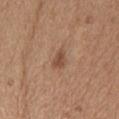Background: The lesion's longest dimension is about 2.5 mm. The tile uses white-light illumination. The lesion-visualizer software estimated a nevus-likeness score of about 75/100 and lesion-presence confidence of about 100/100. A female patient, aged around 70. The lesion is located on the head or neck. Cropped from a whole-body photographic skin survey; the tile spans about 15 mm.